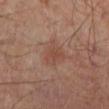biopsy status: imaged on a skin check; not biopsied
patient: male, about 70 years old
diameter: ~3.5 mm (longest diameter)
TBP lesion metrics: a border-irregularity rating of about 3.5/10 and internal color variation of about 2 on a 0–10 scale
image: ~15 mm crop, total-body skin-cancer survey
lighting: cross-polarized illumination
anatomic site: the left lower leg